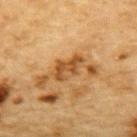The lesion was photographed on a routine skin check and not biopsied; there is no pathology result. A 15 mm crop from a total-body photograph taken for skin-cancer surveillance. Automated tile analysis of the lesion measured a lesion-to-skin contrast of about 7.5 (normalized; higher = more distinct). It also reported a color-variation rating of about 5.5/10 and peripheral color asymmetry of about 2. The lesion is on the upper back. Measured at roughly 4 mm in maximum diameter. The subject is a male approximately 85 years of age or older.